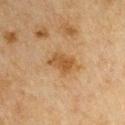Assessment:
Captured during whole-body skin photography for melanoma surveillance; the lesion was not biopsied.
Image and clinical context:
Approximately 3.5 mm at its widest. The tile uses cross-polarized illumination. The lesion is on the right upper arm. The patient is a male roughly 65 years of age. The total-body-photography lesion software estimated a lesion color around L≈42 a*≈17 b*≈33 in CIELAB and a normalized lesion–skin contrast near 7.5. And it measured border irregularity of about 2.5 on a 0–10 scale, a color-variation rating of about 2.5/10, and radial color variation of about 0.5. This image is a 15 mm lesion crop taken from a total-body photograph.